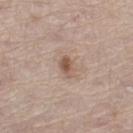Impression:
Imaged during a routine full-body skin examination; the lesion was not biopsied and no histopathology is available.